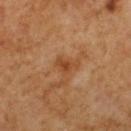The lesion was photographed on a routine skin check and not biopsied; there is no pathology result.
Located on the upper back.
This image is a 15 mm lesion crop taken from a total-body photograph.
This is a cross-polarized tile.
Measured at roughly 2.5 mm in maximum diameter.
The lesion-visualizer software estimated an area of roughly 3.5 mm², an outline eccentricity of about 0.75 (0 = round, 1 = elongated), and two-axis asymmetry of about 0.45.
A male patient, about 60 years old.